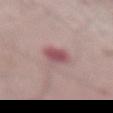follow-up: no biopsy performed (imaged during a skin exam)
imaging modality: ~15 mm tile from a whole-body skin photo
TBP lesion metrics: a footprint of about 7 mm² and an outline eccentricity of about 0.85 (0 = round, 1 = elongated); a mean CIELAB color near L≈53 a*≈24 b*≈17 and a normalized lesion–skin contrast near 8; an automated nevus-likeness rating near 25 out of 100 and lesion-presence confidence of about 100/100
lesion diameter: ≈4.5 mm
patient: male, in their mid-50s
tile lighting: white-light